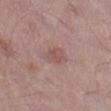Assessment: Recorded during total-body skin imaging; not selected for excision or biopsy. Context: Captured under white-light illumination. A male patient, in their mid-50s. This image is a 15 mm lesion crop taken from a total-body photograph. An algorithmic analysis of the crop reported a lesion area of about 4.5 mm² and two-axis asymmetry of about 0.2. And it measured an average lesion color of about L≈52 a*≈20 b*≈22 (CIELAB), roughly 7 lightness units darker than nearby skin, and a normalized lesion–skin contrast near 5.5. The lesion is on the left thigh.